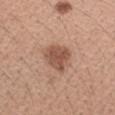This lesion was catalogued during total-body skin photography and was not selected for biopsy.
This image is a 15 mm lesion crop taken from a total-body photograph.
On the right forearm.
A female patient, roughly 45 years of age.